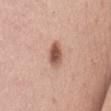The lesion is located on the back. A 15 mm close-up extracted from a 3D total-body photography capture. About 4 mm across. The subject is a female aged 63 to 67.